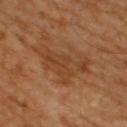{"biopsy_status": "not biopsied; imaged during a skin examination", "patient": {"sex": "male", "age_approx": 65}, "site": "upper back", "image": {"source": "total-body photography crop", "field_of_view_mm": 15}, "lesion_size": {"long_diameter_mm_approx": 7.5}, "automated_metrics": {"area_mm2_approx": 16.0, "eccentricity": 0.9, "nevus_likeness_0_100": 5}, "lighting": "cross-polarized"}